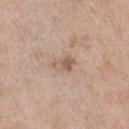No biopsy was performed on this lesion — it was imaged during a full skin examination and was not determined to be concerning.
This image is a 15 mm lesion crop taken from a total-body photograph.
Captured under white-light illumination.
From the right upper arm.
A male patient, aged approximately 65.